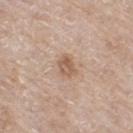Findings:
• follow-up: catalogued during a skin exam; not biopsied
• lesion diameter: ~3 mm (longest diameter)
• lighting: white-light
• image: ~15 mm tile from a whole-body skin photo
• patient: female, aged around 75
• site: the right thigh